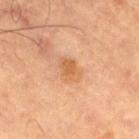Imaged during a routine full-body skin examination; the lesion was not biopsied and no histopathology is available.
The lesion is on the left thigh.
Cropped from a whole-body photographic skin survey; the tile spans about 15 mm.
The tile uses cross-polarized illumination.
A male subject in their mid- to late 60s.